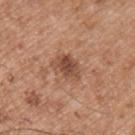Impression: This lesion was catalogued during total-body skin photography and was not selected for biopsy. Clinical summary: This is a white-light tile. Measured at roughly 3.5 mm in maximum diameter. A male subject in their mid-50s. Located on the left upper arm. A close-up tile cropped from a whole-body skin photograph, about 15 mm across.